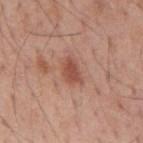Imaged during a routine full-body skin examination; the lesion was not biopsied and no histopathology is available.
Approximately 3.5 mm at its widest.
This is a white-light tile.
The patient is a male aged 58 to 62.
A 15 mm close-up extracted from a 3D total-body photography capture.
On the mid back.
The lesion-visualizer software estimated two-axis asymmetry of about 0.3. The analysis additionally found a classifier nevus-likeness of about 75/100 and a detector confidence of about 100 out of 100 that the crop contains a lesion.